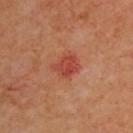Imaged during a routine full-body skin examination; the lesion was not biopsied and no histopathology is available. The recorded lesion diameter is about 3 mm. From the upper back. The tile uses cross-polarized illumination. A 15 mm close-up extracted from a 3D total-body photography capture. The total-body-photography lesion software estimated a footprint of about 5.5 mm² and two-axis asymmetry of about 0.25. And it measured a border-irregularity rating of about 3/10, a color-variation rating of about 3.5/10, and radial color variation of about 1.5. A male subject approximately 65 years of age.